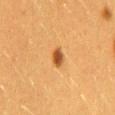Image and clinical context:
The lesion is on the back. Imaged with cross-polarized lighting. The lesion-visualizer software estimated a footprint of about 3.5 mm² and a shape eccentricity near 0.75. The software also gave a lesion color around L≈43 a*≈23 b*≈38 in CIELAB, roughly 13 lightness units darker than nearby skin, and a normalized lesion–skin contrast near 10. The analysis additionally found a border-irregularity rating of about 2/10 and a color-variation rating of about 2.5/10. The analysis additionally found a nevus-likeness score of about 100/100 and a lesion-detection confidence of about 100/100. Measured at roughly 2.5 mm in maximum diameter. The subject is a female roughly 40 years of age. Cropped from a whole-body photographic skin survey; the tile spans about 15 mm.